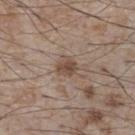| field | value |
|---|---|
| workup | imaged on a skin check; not biopsied |
| lesion size | ≈2.5 mm |
| patient | male, aged 73 to 77 |
| imaging modality | total-body-photography crop, ~15 mm field of view |
| illumination | white-light |
| anatomic site | the chest |
| automated lesion analysis | a lesion area of about 4.5 mm², an outline eccentricity of about 0.4 (0 = round, 1 = elongated), and two-axis asymmetry of about 0.15; a mean CIELAB color near L≈47 a*≈16 b*≈25, a lesion–skin lightness drop of about 10, and a lesion-to-skin contrast of about 7.5 (normalized; higher = more distinct); a nevus-likeness score of about 40/100 and lesion-presence confidence of about 100/100 |